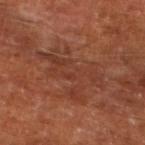Imaged during a routine full-body skin examination; the lesion was not biopsied and no histopathology is available. A roughly 15 mm field-of-view crop from a total-body skin photograph. The total-body-photography lesion software estimated a lesion area of about 20 mm², an outline eccentricity of about 0.8 (0 = round, 1 = elongated), and a shape-asymmetry score of about 0.5 (0 = symmetric). The software also gave a lesion color around L≈38 a*≈24 b*≈30 in CIELAB, roughly 6 lightness units darker than nearby skin, and a lesion-to-skin contrast of about 5 (normalized; higher = more distinct). The software also gave a border-irregularity index near 8.5/10, internal color variation of about 3.5 on a 0–10 scale, and radial color variation of about 1. And it measured a nevus-likeness score of about 0/100 and lesion-presence confidence of about 80/100. Located on the left lower leg. A subject roughly 65 years of age. Captured under cross-polarized illumination.